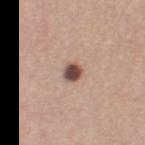Assessment:
The lesion was photographed on a routine skin check and not biopsied; there is no pathology result.
Acquisition and patient details:
A 15 mm crop from a total-body photograph taken for skin-cancer surveillance. The lesion is on the left thigh. An algorithmic analysis of the crop reported two-axis asymmetry of about 0.15. The analysis additionally found border irregularity of about 1 on a 0–10 scale and a peripheral color-asymmetry measure near 1.5. The analysis additionally found an automated nevus-likeness rating near 100 out of 100 and lesion-presence confidence of about 100/100. About 2 mm across. The subject is a female approximately 45 years of age. Imaged with white-light lighting.